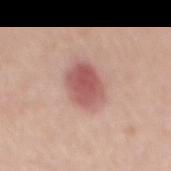<lesion>
  <biopsy_status>not biopsied; imaged during a skin examination</biopsy_status>
  <lesion_size>
    <long_diameter_mm_approx>5.0</long_diameter_mm_approx>
  </lesion_size>
  <site>mid back</site>
  <lighting>white-light</lighting>
  <patient>
    <sex>female</sex>
    <age_approx>60</age_approx>
  </patient>
  <image>
    <source>total-body photography crop</source>
    <field_of_view_mm>15</field_of_view_mm>
  </image>
</lesion>